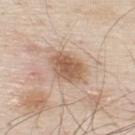This lesion was catalogued during total-body skin photography and was not selected for biopsy. A male subject, roughly 80 years of age. The lesion is located on the upper back. Cropped from a total-body skin-imaging series; the visible field is about 15 mm.